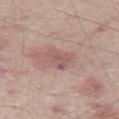<record>
  <biopsy_status>not biopsied; imaged during a skin examination</biopsy_status>
  <image>
    <source>total-body photography crop</source>
    <field_of_view_mm>15</field_of_view_mm>
  </image>
  <site>leg</site>
  <patient>
    <sex>male</sex>
    <age_approx>65</age_approx>
  </patient>
  <lesion_size>
    <long_diameter_mm_approx>3.0</long_diameter_mm_approx>
  </lesion_size>
</record>